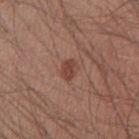Clinical impression: No biopsy was performed on this lesion — it was imaged during a full skin examination and was not determined to be concerning. Image and clinical context: A male patient, aged around 40. Automated tile analysis of the lesion measured a lesion color around L≈43 a*≈23 b*≈26 in CIELAB and about 9 CIELAB-L* units darker than the surrounding skin. Longest diameter approximately 2.5 mm. A 15 mm close-up tile from a total-body photography series done for melanoma screening. Located on the right upper arm. Captured under white-light illumination.